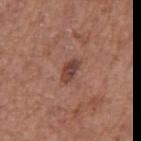- biopsy status · catalogued during a skin exam; not biopsied
- lesion size · about 3 mm
- patient · male, aged 73 to 77
- site · the right upper arm
- imaging modality · ~15 mm crop, total-body skin-cancer survey